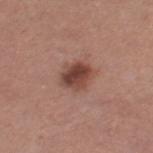Clinical impression:
Part of a total-body skin-imaging series; this lesion was reviewed on a skin check and was not flagged for biopsy.
Background:
Automated tile analysis of the lesion measured an area of roughly 9 mm², an outline eccentricity of about 0.5 (0 = round, 1 = elongated), and a shape-asymmetry score of about 0.2 (0 = symmetric). The analysis additionally found a mean CIELAB color near L≈45 a*≈22 b*≈25, roughly 12 lightness units darker than nearby skin, and a normalized border contrast of about 9. And it measured border irregularity of about 2 on a 0–10 scale and a within-lesion color-variation index near 6/10. About 4 mm across. A region of skin cropped from a whole-body photographic capture, roughly 15 mm wide. The patient is a female aged 28–32. Captured under white-light illumination. The lesion is located on the right thigh.